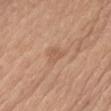Findings:
– biopsy status — catalogued during a skin exam; not biopsied
– image — total-body-photography crop, ~15 mm field of view
– subject — male, about 70 years old
– body site — the left thigh
– illumination — white-light
– automated metrics — a mean CIELAB color near L≈57 a*≈21 b*≈32; a border-irregularity index near 3.5/10; a nevus-likeness score of about 0/100 and lesion-presence confidence of about 100/100
– lesion size — about 2.5 mm